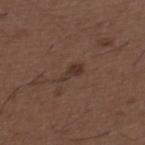Clinical impression:
This lesion was catalogued during total-body skin photography and was not selected for biopsy.
Context:
Longest diameter approximately 3 mm. The lesion-visualizer software estimated an average lesion color of about L≈33 a*≈16 b*≈22 (CIELAB), about 7 CIELAB-L* units darker than the surrounding skin, and a lesion-to-skin contrast of about 7 (normalized; higher = more distinct). From the upper back. A male patient, in their 50s. A region of skin cropped from a whole-body photographic capture, roughly 15 mm wide. This is a white-light tile.